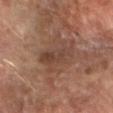Q: What is the anatomic site?
A: the right forearm
Q: How was this image acquired?
A: 15 mm crop, total-body photography
Q: Illumination type?
A: cross-polarized illumination
Q: What are the patient's age and sex?
A: male, in their mid- to late 60s